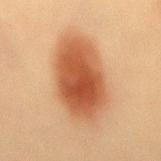A male subject, roughly 60 years of age.
On the mid back.
Imaged with cross-polarized lighting.
Cropped from a whole-body photographic skin survey; the tile spans about 15 mm.